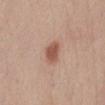Background: A female subject aged 48–52. The lesion's longest dimension is about 3 mm. On the abdomen. This is a white-light tile. A close-up tile cropped from a whole-body skin photograph, about 15 mm across.